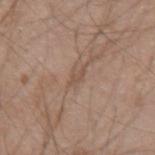The lesion was tiled from a total-body skin photograph and was not biopsied. An algorithmic analysis of the crop reported a lesion color around L≈51 a*≈17 b*≈27 in CIELAB, about 7 CIELAB-L* units darker than the surrounding skin, and a lesion-to-skin contrast of about 5 (normalized; higher = more distinct). The analysis additionally found border irregularity of about 4 on a 0–10 scale and internal color variation of about 0 on a 0–10 scale. A male subject, about 20 years old. A region of skin cropped from a whole-body photographic capture, roughly 15 mm wide. The lesion's longest dimension is about 2.5 mm. From the left upper arm. This is a white-light tile.